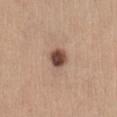Clinical impression: Captured during whole-body skin photography for melanoma surveillance; the lesion was not biopsied. Context: The lesion is located on the abdomen. Captured under white-light illumination. The patient is a female roughly 45 years of age. This image is a 15 mm lesion crop taken from a total-body photograph. The recorded lesion diameter is about 2.5 mm.